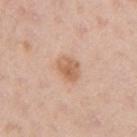{
  "biopsy_status": "not biopsied; imaged during a skin examination",
  "automated_metrics": {
    "border_irregularity_0_10": 1.5,
    "color_variation_0_10": 3.5,
    "peripheral_color_asymmetry": 1.0
  },
  "site": "right upper arm",
  "lesion_size": {
    "long_diameter_mm_approx": 3.0
  },
  "image": {
    "source": "total-body photography crop",
    "field_of_view_mm": 15
  },
  "patient": {
    "sex": "female",
    "age_approx": 40
  }
}